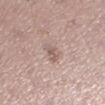Captured during whole-body skin photography for melanoma surveillance; the lesion was not biopsied. The subject is a female aged approximately 35. Located on the left lower leg. Captured under white-light illumination. Longest diameter approximately 3 mm. A 15 mm crop from a total-body photograph taken for skin-cancer surveillance.